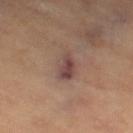Background: From the right thigh. A 15 mm close-up tile from a total-body photography series done for melanoma screening. The tile uses cross-polarized illumination. Measured at roughly 4 mm in maximum diameter. An algorithmic analysis of the crop reported an average lesion color of about L≈46 a*≈19 b*≈22 (CIELAB), a lesion–skin lightness drop of about 10, and a normalized border contrast of about 8.5. A female patient, about 70 years old.